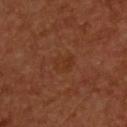Recorded during total-body skin imaging; not selected for excision or biopsy.
Approximately 3 mm at its widest.
A region of skin cropped from a whole-body photographic capture, roughly 15 mm wide.
The lesion is located on the upper back.
Imaged with cross-polarized lighting.
The total-body-photography lesion software estimated an area of roughly 5 mm², a shape eccentricity near 0.65, and two-axis asymmetry of about 0.3. It also reported about 3 CIELAB-L* units darker than the surrounding skin. The analysis additionally found a border-irregularity rating of about 3/10, a within-lesion color-variation index near 2/10, and peripheral color asymmetry of about 0.5.
A male patient about 55 years old.